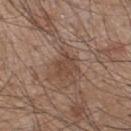Q: Is there a histopathology result?
A: no biopsy performed (imaged during a skin exam)
Q: How was the tile lit?
A: white-light
Q: What kind of image is this?
A: 15 mm crop, total-body photography
Q: What are the patient's age and sex?
A: male, about 45 years old
Q: Lesion location?
A: the upper back